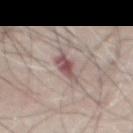<lesion>
  <biopsy_status>not biopsied; imaged during a skin examination</biopsy_status>
</lesion>